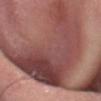Captured during whole-body skin photography for melanoma surveillance; the lesion was not biopsied.
The patient is a male in their mid- to late 40s.
The lesion is on the head or neck.
A close-up tile cropped from a whole-body skin photograph, about 15 mm across.